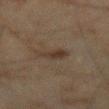notes — no biopsy performed (imaged during a skin exam) | lighting — cross-polarized illumination | imaging modality — ~15 mm tile from a whole-body skin photo | size — ≈3 mm | site — the right forearm | patient — male, about 45 years old | automated metrics — border irregularity of about 4 on a 0–10 scale, a within-lesion color-variation index near 2.5/10, and radial color variation of about 0.5.